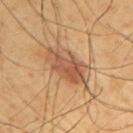Clinical impression:
This lesion was catalogued during total-body skin photography and was not selected for biopsy.
Clinical summary:
Automated image analysis of the tile measured a mean CIELAB color near L≈55 a*≈24 b*≈35 and roughly 11 lightness units darker than nearby skin. Captured under cross-polarized illumination. A close-up tile cropped from a whole-body skin photograph, about 15 mm across. Approximately 5.5 mm at its widest. Located on the right upper arm. A male subject, aged approximately 65.